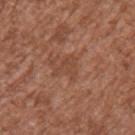This lesion was catalogued during total-body skin photography and was not selected for biopsy. Automated tile analysis of the lesion measured about 6 CIELAB-L* units darker than the surrounding skin and a normalized border contrast of about 5. And it measured border irregularity of about 7.5 on a 0–10 scale, a color-variation rating of about 1.5/10, and a peripheral color-asymmetry measure near 0.5. On the left upper arm. A region of skin cropped from a whole-body photographic capture, roughly 15 mm wide. Longest diameter approximately 3 mm. The patient is a male aged 43 to 47.